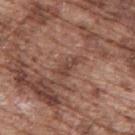{
  "biopsy_status": "not biopsied; imaged during a skin examination",
  "image": {
    "source": "total-body photography crop",
    "field_of_view_mm": 15
  },
  "automated_metrics": {
    "eccentricity": 0.9,
    "cielab_L": 43,
    "cielab_a": 20,
    "cielab_b": 25,
    "vs_skin_darker_L": 8.0,
    "vs_skin_contrast_norm": 6.0
  },
  "lesion_size": {
    "long_diameter_mm_approx": 3.0
  },
  "patient": {
    "sex": "male",
    "age_approx": 75
  },
  "site": "upper back",
  "lighting": "white-light"
}